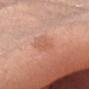Clinical impression:
Part of a total-body skin-imaging series; this lesion was reviewed on a skin check and was not flagged for biopsy.
Background:
The lesion's longest dimension is about 3 mm. An algorithmic analysis of the crop reported a border-irregularity rating of about 2/10 and a color-variation rating of about 3/10. The software also gave a detector confidence of about 100 out of 100 that the crop contains a lesion. From the head or neck. Cropped from a total-body skin-imaging series; the visible field is about 15 mm. Imaged with white-light lighting. A female subject roughly 50 years of age.